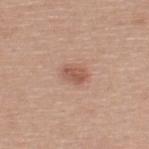follow-up: no biopsy performed (imaged during a skin exam)
TBP lesion metrics: an area of roughly 4 mm², an outline eccentricity of about 0.75 (0 = round, 1 = elongated), and two-axis asymmetry of about 0.15; an average lesion color of about L≈55 a*≈22 b*≈29 (CIELAB), roughly 10 lightness units darker than nearby skin, and a normalized lesion–skin contrast near 7; a nevus-likeness score of about 80/100 and lesion-presence confidence of about 100/100
diameter: about 2.5 mm
image source: total-body-photography crop, ~15 mm field of view
body site: the upper back
subject: male, in their 40s
illumination: white-light